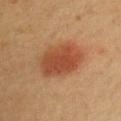{"biopsy_status": "not biopsied; imaged during a skin examination", "site": "chest", "patient": {"sex": "male", "age_approx": 40}, "lighting": "cross-polarized", "image": {"source": "total-body photography crop", "field_of_view_mm": 15}}